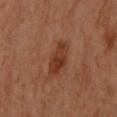notes: total-body-photography surveillance lesion; no biopsy | patient: female, aged approximately 60 | location: the left arm | size: ≈4.5 mm | illumination: cross-polarized illumination | imaging modality: total-body-photography crop, ~15 mm field of view | TBP lesion metrics: border irregularity of about 2.5 on a 0–10 scale, a within-lesion color-variation index near 4/10, and a peripheral color-asymmetry measure near 1.5.